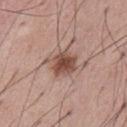This image is a 15 mm lesion crop taken from a total-body photograph.
The subject is a male aged approximately 55.
The tile uses white-light illumination.
The recorded lesion diameter is about 4 mm.
The lesion is on the abdomen.
The lesion-visualizer software estimated a border-irregularity index near 2.5/10, internal color variation of about 4 on a 0–10 scale, and radial color variation of about 1.